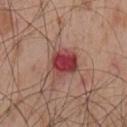Findings:
* notes — catalogued during a skin exam; not biopsied
* patient — male, about 55 years old
* site — the chest
* size — ~3.5 mm (longest diameter)
* image source — ~15 mm crop, total-body skin-cancer survey
* tile lighting — white-light illumination